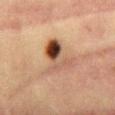  biopsy_status: not biopsied; imaged during a skin examination
  image:
    source: total-body photography crop
    field_of_view_mm: 15
  site: abdomen
  automated_metrics:
    area_mm2_approx: 12.0
    eccentricity: 0.65
    shape_asymmetry: 0.4
    cielab_L: 43
    cielab_a: 18
    cielab_b: 27
    vs_skin_darker_L: 12.0
    vs_skin_contrast_norm: 9.5
  patient:
    sex: female
    age_approx: 40
  lesion_size:
    long_diameter_mm_approx: 4.5